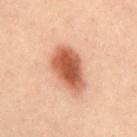- notes — no biopsy performed (imaged during a skin exam)
- body site — the front of the torso
- imaging modality — 15 mm crop, total-body photography
- diameter — about 5.5 mm
- patient — male, approximately 40 years of age
- illumination — cross-polarized